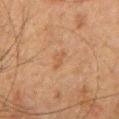<lesion>
<biopsy_status>not biopsied; imaged during a skin examination</biopsy_status>
<lesion_size>
  <long_diameter_mm_approx>2.5</long_diameter_mm_approx>
</lesion_size>
<lighting>cross-polarized</lighting>
<automated_metrics>
  <eccentricity>0.85</eccentricity>
  <shape_asymmetry>0.5</shape_asymmetry>
</automated_metrics>
<site>chest</site>
<patient>
  <sex>male</sex>
  <age_approx>50</age_approx>
</patient>
<image>
  <source>total-body photography crop</source>
  <field_of_view_mm>15</field_of_view_mm>
</image>
</lesion>